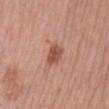biopsy status: catalogued during a skin exam; not biopsied
image: 15 mm crop, total-body photography
anatomic site: the right lower leg
subject: female, aged 63–67